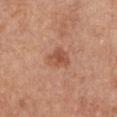Q: Is there a histopathology result?
A: no biopsy performed (imaged during a skin exam)
Q: What are the patient's age and sex?
A: female, aged 53–57
Q: What did automated image analysis measure?
A: a lesion area of about 5.5 mm², an outline eccentricity of about 0.55 (0 = round, 1 = elongated), and two-axis asymmetry of about 0.3; an average lesion color of about L≈52 a*≈25 b*≈32 (CIELAB), about 9 CIELAB-L* units darker than the surrounding skin, and a normalized border contrast of about 6.5
Q: How was the tile lit?
A: white-light
Q: What is the anatomic site?
A: the chest
Q: How was this image acquired?
A: ~15 mm tile from a whole-body skin photo